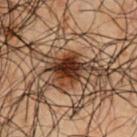| feature | finding |
|---|---|
| notes | imaged on a skin check; not biopsied |
| imaging modality | ~15 mm crop, total-body skin-cancer survey |
| size | ≈3 mm |
| location | the chest |
| automated metrics | a lesion area of about 6 mm², a shape eccentricity near 0.55, and a shape-asymmetry score of about 0.3 (0 = symmetric); an average lesion color of about L≈21 a*≈17 b*≈22 (CIELAB); border irregularity of about 3.5 on a 0–10 scale |
| subject | male, aged around 50 |